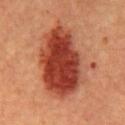Notes:
– follow-up: catalogued during a skin exam; not biopsied
– image source: total-body-photography crop, ~15 mm field of view
– patient: male, aged 63–67
– anatomic site: the abdomen